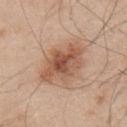Assessment: Recorded during total-body skin imaging; not selected for excision or biopsy. Background: A lesion tile, about 15 mm wide, cut from a 3D total-body photograph. On the upper back. Imaged with white-light lighting. A male patient aged approximately 55. Measured at roughly 7 mm in maximum diameter.